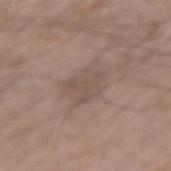Clinical impression:
Recorded during total-body skin imaging; not selected for excision or biopsy.
Clinical summary:
This is a white-light tile. Located on the right forearm. A 15 mm crop from a total-body photograph taken for skin-cancer surveillance. Automated tile analysis of the lesion measured a color-variation rating of about 2/10 and peripheral color asymmetry of about 0.5. A male subject aged 58 to 62. Longest diameter approximately 4.5 mm.